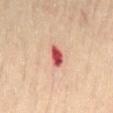The lesion was photographed on a routine skin check and not biopsied; there is no pathology result. The lesion is located on the lower back. Approximately 3 mm at its widest. A male subject, approximately 70 years of age. The total-body-photography lesion software estimated a lesion color around L≈54 a*≈34 b*≈28 in CIELAB, a lesion–skin lightness drop of about 17, and a normalized lesion–skin contrast near 11. And it measured border irregularity of about 2.5 on a 0–10 scale, a color-variation rating of about 4/10, and a peripheral color-asymmetry measure near 1.5. A 15 mm crop from a total-body photograph taken for skin-cancer surveillance.